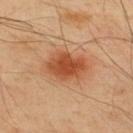Q: Was a biopsy performed?
A: total-body-photography surveillance lesion; no biopsy
Q: What lighting was used for the tile?
A: cross-polarized illumination
Q: What is the imaging modality?
A: ~15 mm tile from a whole-body skin photo
Q: Patient demographics?
A: male, roughly 50 years of age
Q: What did automated image analysis measure?
A: a classifier nevus-likeness of about 100/100
Q: What is the anatomic site?
A: the upper back